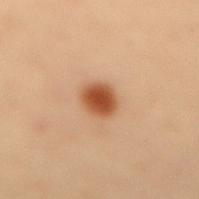Assessment: Part of a total-body skin-imaging series; this lesion was reviewed on a skin check and was not flagged for biopsy. Image and clinical context: The recorded lesion diameter is about 3 mm. A female subject about 50 years old. This is a cross-polarized tile. This image is a 15 mm lesion crop taken from a total-body photograph. The lesion is located on the mid back.